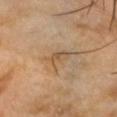notes = catalogued during a skin exam; not biopsied | diameter = ≈3 mm | patient = male, approximately 60 years of age | image source = 15 mm crop, total-body photography | site = the head or neck | illumination = cross-polarized.